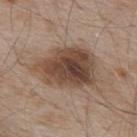Q: Was this lesion biopsied?
A: catalogued during a skin exam; not biopsied
Q: Automated lesion metrics?
A: a footprint of about 26 mm² and two-axis asymmetry of about 0.2; a color-variation rating of about 7/10 and a peripheral color-asymmetry measure near 2; an automated nevus-likeness rating near 75 out of 100 and a lesion-detection confidence of about 100/100
Q: Patient demographics?
A: male, roughly 55 years of age
Q: What is the lesion's diameter?
A: ~7 mm (longest diameter)
Q: What is the anatomic site?
A: the upper back
Q: What is the imaging modality?
A: ~15 mm crop, total-body skin-cancer survey
Q: How was the tile lit?
A: white-light illumination